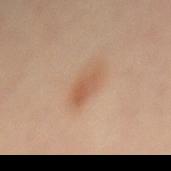Impression: Captured during whole-body skin photography for melanoma surveillance; the lesion was not biopsied. Clinical summary: Approximately 4.5 mm at its widest. The lesion is located on the mid back. A female patient aged around 45. Imaged with cross-polarized lighting. Cropped from a whole-body photographic skin survey; the tile spans about 15 mm. Automated image analysis of the tile measured an area of roughly 6.5 mm² and a shape-asymmetry score of about 0.25 (0 = symmetric). The software also gave a lesion color around L≈56 a*≈20 b*≈32 in CIELAB. The software also gave a within-lesion color-variation index near 4/10 and peripheral color asymmetry of about 1.5.